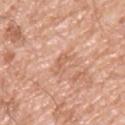Imaged during a routine full-body skin examination; the lesion was not biopsied and no histopathology is available. Measured at roughly 2.5 mm in maximum diameter. The total-body-photography lesion software estimated an average lesion color of about L≈62 a*≈23 b*≈33 (CIELAB), a lesion–skin lightness drop of about 8, and a normalized border contrast of about 5.5. The lesion is on the upper back. The tile uses white-light illumination. The subject is a male aged approximately 55. A region of skin cropped from a whole-body photographic capture, roughly 15 mm wide.